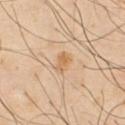follow-up: no biopsy performed (imaged during a skin exam)
patient: male, aged approximately 45
acquisition: ~15 mm crop, total-body skin-cancer survey
location: the chest